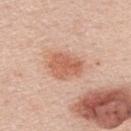A lesion tile, about 15 mm wide, cut from a 3D total-body photograph.
A male subject, aged approximately 60.
The lesion's longest dimension is about 4.5 mm.
On the upper back.